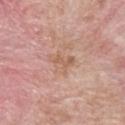Impression:
Part of a total-body skin-imaging series; this lesion was reviewed on a skin check and was not flagged for biopsy.
Acquisition and patient details:
The lesion is located on the upper back. A male subject approximately 60 years of age. This is a white-light tile. A 15 mm crop from a total-body photograph taken for skin-cancer surveillance.